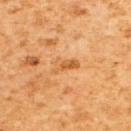Q: Was this lesion biopsied?
A: catalogued during a skin exam; not biopsied
Q: Who is the patient?
A: male, approximately 60 years of age
Q: What is the anatomic site?
A: the back
Q: How large is the lesion?
A: ≈3.5 mm
Q: What kind of image is this?
A: ~15 mm tile from a whole-body skin photo